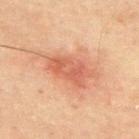Imaged during a routine full-body skin examination; the lesion was not biopsied and no histopathology is available. A male patient, approximately 45 years of age. A close-up tile cropped from a whole-body skin photograph, about 15 mm across. The lesion is located on the upper back.